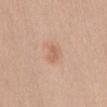Clinical impression: No biopsy was performed on this lesion — it was imaged during a full skin examination and was not determined to be concerning. Image and clinical context: A female patient, aged approximately 45. Located on the abdomen. Cropped from a total-body skin-imaging series; the visible field is about 15 mm.